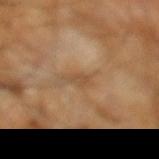imaging modality = ~15 mm tile from a whole-body skin photo; subject = male, aged 58 to 62; tile lighting = cross-polarized illumination; location = the left lower leg; diameter = ≈3 mm.